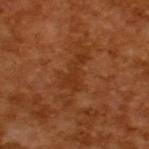Imaged during a routine full-body skin examination; the lesion was not biopsied and no histopathology is available. About 4 mm across. A male subject, aged approximately 65. Captured under cross-polarized illumination. Automated image analysis of the tile measured a normalized border contrast of about 6. The analysis additionally found a detector confidence of about 100 out of 100 that the crop contains a lesion. A close-up tile cropped from a whole-body skin photograph, about 15 mm across.